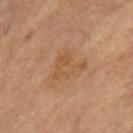subject: female, approximately 70 years of age
image-analysis metrics: an area of roughly 8 mm², an outline eccentricity of about 0.6 (0 = round, 1 = elongated), and two-axis asymmetry of about 0.6; a lesion color around L≈50 a*≈19 b*≈35 in CIELAB and about 6 CIELAB-L* units darker than the surrounding skin
lighting: cross-polarized
lesion diameter: about 4.5 mm
acquisition: total-body-photography crop, ~15 mm field of view
anatomic site: the right lower leg